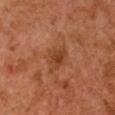Clinical impression: The lesion was tiled from a total-body skin photograph and was not biopsied. Acquisition and patient details: A female subject about 60 years old. Imaged with cross-polarized lighting. The total-body-photography lesion software estimated an area of roughly 6 mm², an outline eccentricity of about 0.55 (0 = round, 1 = elongated), and a symmetry-axis asymmetry near 0.35. The analysis additionally found an average lesion color of about L≈36 a*≈23 b*≈32 (CIELAB) and a lesion-to-skin contrast of about 6.5 (normalized; higher = more distinct). Located on the chest. A 15 mm close-up tile from a total-body photography series done for melanoma screening. Measured at roughly 3 mm in maximum diameter.